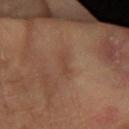site — the arm | patient — female, aged 68–72 | illumination — cross-polarized illumination | acquisition — total-body-photography crop, ~15 mm field of view.